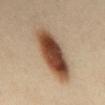{
  "biopsy_status": "not biopsied; imaged during a skin examination",
  "lighting": "cross-polarized",
  "site": "back",
  "patient": {
    "sex": "female",
    "age_approx": 40
  },
  "image": {
    "source": "total-body photography crop",
    "field_of_view_mm": 15
  },
  "lesion_size": {
    "long_diameter_mm_approx": 8.0
  }
}